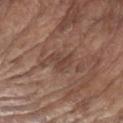Impression:
Captured during whole-body skin photography for melanoma surveillance; the lesion was not biopsied.
Context:
Automated tile analysis of the lesion measured a normalized lesion–skin contrast near 6. It also reported border irregularity of about 5 on a 0–10 scale, a color-variation rating of about 2/10, and a peripheral color-asymmetry measure near 0.5. It also reported an automated nevus-likeness rating near 0 out of 100 and lesion-presence confidence of about 100/100. Located on the left upper arm. A 15 mm close-up extracted from a 3D total-body photography capture. This is a white-light tile. The subject is a male approximately 80 years of age. The lesion's longest dimension is about 3 mm.